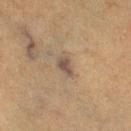Findings:
– tile lighting — cross-polarized illumination
– body site — the leg
– subject — female, aged 53 to 57
– image source — 15 mm crop, total-body photography